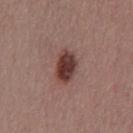workup: imaged on a skin check; not biopsied
subject: male, about 40 years old
lesion diameter: ~4 mm (longest diameter)
automated metrics: border irregularity of about 2 on a 0–10 scale, internal color variation of about 5.5 on a 0–10 scale, and a peripheral color-asymmetry measure near 1.5
lighting: white-light
image: ~15 mm tile from a whole-body skin photo
anatomic site: the chest